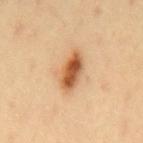The lesion is located on the mid back. The tile uses cross-polarized illumination. A male subject, aged approximately 40. An algorithmic analysis of the crop reported an average lesion color of about L≈58 a*≈24 b*≈40 (CIELAB). And it measured a color-variation rating of about 6/10 and a peripheral color-asymmetry measure near 2. A 15 mm close-up extracted from a 3D total-body photography capture. Longest diameter approximately 5 mm.